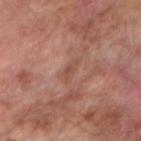This lesion was catalogued during total-body skin photography and was not selected for biopsy. The lesion is located on the right forearm. Imaged with cross-polarized lighting. A 15 mm close-up extracted from a 3D total-body photography capture. The lesion's longest dimension is about 3 mm. The subject is a male roughly 65 years of age.